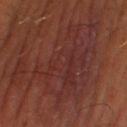Captured during whole-body skin photography for melanoma surveillance; the lesion was not biopsied. Captured under cross-polarized illumination. The lesion's longest dimension is about 14 mm. Automated tile analysis of the lesion measured a border-irregularity index near 9.5/10 and peripheral color asymmetry of about 1.5. The analysis additionally found a detector confidence of about 50 out of 100 that the crop contains a lesion. Located on the right lower leg. A male patient approximately 55 years of age. A 15 mm close-up tile from a total-body photography series done for melanoma screening.